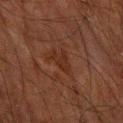notes: total-body-photography surveillance lesion; no biopsy
acquisition: total-body-photography crop, ~15 mm field of view
patient: male, in their mid- to late 60s
automated lesion analysis: an area of roughly 4 mm², an outline eccentricity of about 0.7 (0 = round, 1 = elongated), and two-axis asymmetry of about 0.45
anatomic site: the left forearm
size: ~3 mm (longest diameter)
illumination: cross-polarized illumination